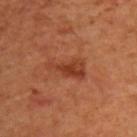• biopsy status: catalogued during a skin exam; not biopsied
• location: the back
• lesion size: about 4.5 mm
• tile lighting: cross-polarized
• subject: male, in their 70s
• image-analysis metrics: a footprint of about 7.5 mm², a shape eccentricity near 0.9, and a shape-asymmetry score of about 0.5 (0 = symmetric); a lesion color around L≈39 a*≈28 b*≈34 in CIELAB, roughly 8 lightness units darker than nearby skin, and a normalized border contrast of about 7
• acquisition: total-body-photography crop, ~15 mm field of view